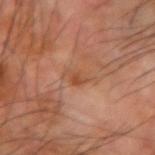Assessment: This lesion was catalogued during total-body skin photography and was not selected for biopsy. Context: A male patient, roughly 70 years of age. Measured at roughly 3.5 mm in maximum diameter. An algorithmic analysis of the crop reported an area of roughly 4.5 mm², a shape eccentricity near 0.85, and two-axis asymmetry of about 0.25. It also reported a border-irregularity rating of about 3/10, internal color variation of about 3.5 on a 0–10 scale, and radial color variation of about 1. The lesion is on the left forearm. A lesion tile, about 15 mm wide, cut from a 3D total-body photograph.